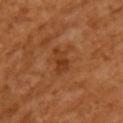Case summary:
- biopsy status: imaged on a skin check; not biopsied
- image source: 15 mm crop, total-body photography
- site: the left upper arm
- tile lighting: cross-polarized illumination
- patient: female, aged 53–57
- automated lesion analysis: a footprint of about 5 mm², a shape eccentricity near 0.8, and two-axis asymmetry of about 0.35; a lesion color around L≈39 a*≈26 b*≈38 in CIELAB, roughly 8 lightness units darker than nearby skin, and a normalized lesion–skin contrast near 6.5; a nevus-likeness score of about 25/100
- size: about 3 mm